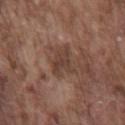Q: Was a biopsy performed?
A: catalogued during a skin exam; not biopsied
Q: How was this image acquired?
A: 15 mm crop, total-body photography
Q: Who is the patient?
A: male, in their mid- to late 70s
Q: Illumination type?
A: white-light illumination
Q: What did automated image analysis measure?
A: a lesion area of about 7 mm², a shape eccentricity near 0.25, and a shape-asymmetry score of about 0.35 (0 = symmetric); roughly 8 lightness units darker than nearby skin and a normalized lesion–skin contrast near 6.5
Q: How large is the lesion?
A: ~3 mm (longest diameter)
Q: Lesion location?
A: the front of the torso